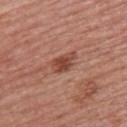The lesion was tiled from a total-body skin photograph and was not biopsied.
Automated image analysis of the tile measured a lesion area of about 5.5 mm², an eccentricity of roughly 0.8, and two-axis asymmetry of about 0.25. And it measured a mean CIELAB color near L≈46 a*≈26 b*≈29 and a normalized border contrast of about 8. It also reported a border-irregularity rating of about 3/10, a color-variation rating of about 3.5/10, and peripheral color asymmetry of about 1. It also reported an automated nevus-likeness rating near 95 out of 100 and lesion-presence confidence of about 100/100.
On the upper back.
The tile uses white-light illumination.
A 15 mm close-up tile from a total-body photography series done for melanoma screening.
A female subject approximately 55 years of age.